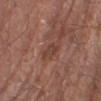This lesion was catalogued during total-body skin photography and was not selected for biopsy.
Measured at roughly 3 mm in maximum diameter.
This is a white-light tile.
From the right lower leg.
A 15 mm crop from a total-body photograph taken for skin-cancer surveillance.
The patient is a male aged around 80.